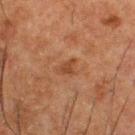| key | value |
|---|---|
| workup | catalogued during a skin exam; not biopsied |
| diameter | about 2.5 mm |
| patient | male, aged approximately 50 |
| tile lighting | cross-polarized illumination |
| image source | ~15 mm crop, total-body skin-cancer survey |
| body site | the upper back |